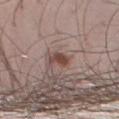follow-up=catalogued during a skin exam; not biopsied
imaging modality=~15 mm crop, total-body skin-cancer survey
size=≈2.5 mm
subject=male, in their mid-30s
anatomic site=the leg